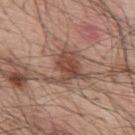Clinical impression: Imaged during a routine full-body skin examination; the lesion was not biopsied and no histopathology is available. Acquisition and patient details: On the mid back. A 15 mm crop from a total-body photograph taken for skin-cancer surveillance. A male patient in their mid- to late 50s.